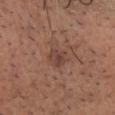- workup · catalogued during a skin exam; not biopsied
- subject · male, aged approximately 65
- imaging modality · total-body-photography crop, ~15 mm field of view
- anatomic site · the head or neck